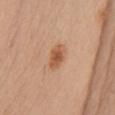No biopsy was performed on this lesion — it was imaged during a full skin examination and was not determined to be concerning.
A region of skin cropped from a whole-body photographic capture, roughly 15 mm wide.
The subject is a male approximately 40 years of age.
The lesion is on the chest.